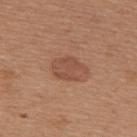Longest diameter approximately 4 mm. The total-body-photography lesion software estimated an outline eccentricity of about 0.7 (0 = round, 1 = elongated) and a shape-asymmetry score of about 0.3 (0 = symmetric). And it measured a mean CIELAB color near L≈49 a*≈23 b*≈30, about 8 CIELAB-L* units darker than the surrounding skin, and a normalized border contrast of about 6. The software also gave a classifier nevus-likeness of about 65/100. The lesion is on the back. A female subject, in their mid-50s. Captured under white-light illumination. Cropped from a total-body skin-imaging series; the visible field is about 15 mm.